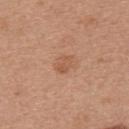Imaged during a routine full-body skin examination; the lesion was not biopsied and no histopathology is available.
On the upper back.
Imaged with white-light lighting.
A female patient in their mid-30s.
A lesion tile, about 15 mm wide, cut from a 3D total-body photograph.
The lesion's longest dimension is about 2.5 mm.